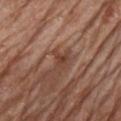Impression: This lesion was catalogued during total-body skin photography and was not selected for biopsy. Image and clinical context: On the chest. The patient is a male aged 78 to 82. The total-body-photography lesion software estimated an area of roughly 4 mm², an eccentricity of roughly 0.7, and two-axis asymmetry of about 0.55. And it measured a border-irregularity index near 7/10 and a within-lesion color-variation index near 3/10. The software also gave lesion-presence confidence of about 90/100. Measured at roughly 3 mm in maximum diameter. A 15 mm crop from a total-body photograph taken for skin-cancer surveillance.